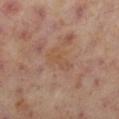This lesion was catalogued during total-body skin photography and was not selected for biopsy. From the right lower leg. This image is a 15 mm lesion crop taken from a total-body photograph. A female subject about 55 years old.